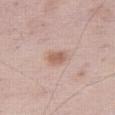The lesion was photographed on a routine skin check and not biopsied; there is no pathology result.
The lesion's longest dimension is about 2.5 mm.
Cropped from a whole-body photographic skin survey; the tile spans about 15 mm.
Located on the right thigh.
A male patient, roughly 60 years of age.
The tile uses white-light illumination.
Automated image analysis of the tile measured roughly 10 lightness units darker than nearby skin and a normalized lesion–skin contrast near 7.